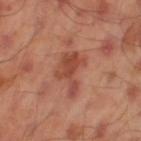follow-up=total-body-photography surveillance lesion; no biopsy | image=~15 mm tile from a whole-body skin photo | subject=male, aged 43 to 47 | anatomic site=the leg.